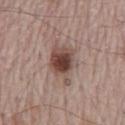workup: imaged on a skin check; not biopsied
patient: male, aged 63–67
diameter: ~5 mm (longest diameter)
tile lighting: white-light
automated metrics: a lesion area of about 12 mm² and an eccentricity of roughly 0.75; a lesion color around L≈46 a*≈18 b*≈23 in CIELAB, roughly 14 lightness units darker than nearby skin, and a lesion-to-skin contrast of about 10 (normalized; higher = more distinct); a classifier nevus-likeness of about 100/100 and a detector confidence of about 100 out of 100 that the crop contains a lesion
body site: the back
imaging modality: 15 mm crop, total-body photography